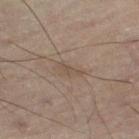Recorded during total-body skin imaging; not selected for excision or biopsy.
A male subject, aged approximately 60.
A lesion tile, about 15 mm wide, cut from a 3D total-body photograph.
From the left thigh.
Automated image analysis of the tile measured a within-lesion color-variation index near 0/10 and peripheral color asymmetry of about 0. It also reported an automated nevus-likeness rating near 0 out of 100.
Captured under cross-polarized illumination.
About 3 mm across.